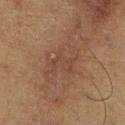Findings:
– biopsy status — total-body-photography surveillance lesion; no biopsy
– illumination — cross-polarized illumination
– anatomic site — the right lower leg
– image source — 15 mm crop, total-body photography
– patient — male, aged around 60
– lesion size — ≈5.5 mm